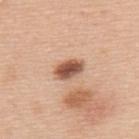Recorded during total-body skin imaging; not selected for excision or biopsy. The lesion is on the upper back. Imaged with white-light lighting. A 15 mm close-up tile from a total-body photography series done for melanoma screening. A male subject, in their 50s. The recorded lesion diameter is about 3.5 mm.